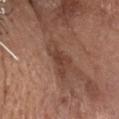The lesion was photographed on a routine skin check and not biopsied; there is no pathology result.
From the head or neck.
Cropped from a whole-body photographic skin survey; the tile spans about 15 mm.
This is a white-light tile.
The patient is a male aged 73 to 77.
The recorded lesion diameter is about 5 mm.
The total-body-photography lesion software estimated a border-irregularity index near 6/10 and a color-variation rating of about 2.5/10. The analysis additionally found a nevus-likeness score of about 5/100 and lesion-presence confidence of about 95/100.